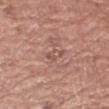Case summary:
* workup: catalogued during a skin exam; not biopsied